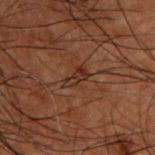Assessment:
Imaged during a routine full-body skin examination; the lesion was not biopsied and no histopathology is available.
Background:
From the back. Automated tile analysis of the lesion measured a mean CIELAB color near L≈22 a*≈17 b*≈22, about 6 CIELAB-L* units darker than the surrounding skin, and a normalized lesion–skin contrast near 7. The analysis additionally found a border-irregularity rating of about 3.5/10, a color-variation rating of about 1/10, and peripheral color asymmetry of about 0. Cropped from a whole-body photographic skin survey; the tile spans about 15 mm. A male subject aged 48 to 52. About 3 mm across.